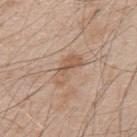The lesion was tiled from a total-body skin photograph and was not biopsied. From the upper back. The recorded lesion diameter is about 4.5 mm. Automated image analysis of the tile measured border irregularity of about 4 on a 0–10 scale and radial color variation of about 1. The software also gave a nevus-likeness score of about 0/100. A roughly 15 mm field-of-view crop from a total-body skin photograph. The subject is a male aged around 50.